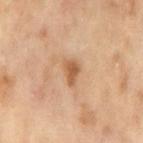Assessment:
This lesion was catalogued during total-body skin photography and was not selected for biopsy.
Context:
A female patient, approximately 50 years of age. The lesion is on the left thigh. This image is a 15 mm lesion crop taken from a total-body photograph.